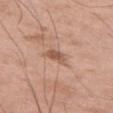Case summary:
* follow-up · imaged on a skin check; not biopsied
* lesion diameter · about 2.5 mm
* imaging modality · ~15 mm crop, total-body skin-cancer survey
* tile lighting · white-light
* subject · male, about 50 years old
* body site · the left thigh